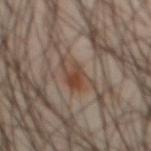Part of a total-body skin-imaging series; this lesion was reviewed on a skin check and was not flagged for biopsy.
A close-up tile cropped from a whole-body skin photograph, about 15 mm across.
The patient is a male aged approximately 45.
Longest diameter approximately 4 mm.
On the abdomen.
Imaged with cross-polarized lighting.